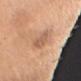Q: Was this lesion biopsied?
A: total-body-photography surveillance lesion; no biopsy
Q: Lesion location?
A: the right upper arm
Q: Automated lesion metrics?
A: a footprint of about 5 mm²; a border-irregularity rating of about 3.5/10 and peripheral color asymmetry of about 0.5; a nevus-likeness score of about 0/100 and lesion-presence confidence of about 100/100
Q: Patient demographics?
A: female, roughly 55 years of age
Q: How large is the lesion?
A: ~3 mm (longest diameter)
Q: How was this image acquired?
A: ~15 mm crop, total-body skin-cancer survey
Q: How was the tile lit?
A: white-light